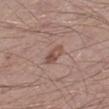• follow-up · total-body-photography surveillance lesion; no biopsy
• location · the leg
• image · ~15 mm tile from a whole-body skin photo
• subject · male, aged 38–42